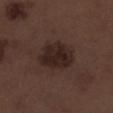The lesion is on the right lower leg.
This image is a 15 mm lesion crop taken from a total-body photograph.
A male subject, in their 70s.